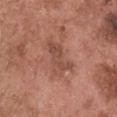Recorded during total-body skin imaging; not selected for excision or biopsy. Automated image analysis of the tile measured a footprint of about 9.5 mm² and two-axis asymmetry of about 0.35. The analysis additionally found an average lesion color of about L≈49 a*≈23 b*≈28 (CIELAB), a lesion–skin lightness drop of about 8, and a normalized border contrast of about 6. The analysis additionally found border irregularity of about 5 on a 0–10 scale, internal color variation of about 3 on a 0–10 scale, and peripheral color asymmetry of about 1. The analysis additionally found an automated nevus-likeness rating near 0 out of 100 and lesion-presence confidence of about 100/100. A close-up tile cropped from a whole-body skin photograph, about 15 mm across. The lesion is located on the head or neck. Imaged with white-light lighting. A male subject, roughly 50 years of age.